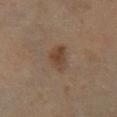| field | value |
|---|---|
| biopsy status | catalogued during a skin exam; not biopsied |
| image source | total-body-photography crop, ~15 mm field of view |
| lesion size | about 3.5 mm |
| location | the leg |
| lighting | cross-polarized |
| patient | male, aged 53–57 |